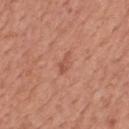Notes:
• notes: total-body-photography surveillance lesion; no biopsy
• anatomic site: the back
• subject: male, approximately 55 years of age
• imaging modality: 15 mm crop, total-body photography